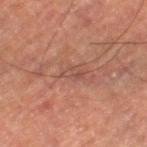This lesion was catalogued during total-body skin photography and was not selected for biopsy. A region of skin cropped from a whole-body photographic capture, roughly 15 mm wide. The lesion is on the left thigh. An algorithmic analysis of the crop reported a lesion area of about 2.5 mm², a shape eccentricity near 0.9, and two-axis asymmetry of about 0.3. And it measured about 6 CIELAB-L* units darker than the surrounding skin and a normalized lesion–skin contrast near 5.5. This is a cross-polarized tile. A male subject in their mid- to late 50s.